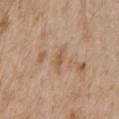{
  "biopsy_status": "not biopsied; imaged during a skin examination",
  "site": "chest",
  "image": {
    "source": "total-body photography crop",
    "field_of_view_mm": 15
  },
  "patient": {
    "sex": "male",
    "age_approx": 65
  }
}